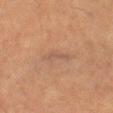Q: Was a biopsy performed?
A: catalogued during a skin exam; not biopsied
Q: Lesion location?
A: the leg
Q: Who is the patient?
A: male, about 60 years old
Q: What is the imaging modality?
A: 15 mm crop, total-body photography